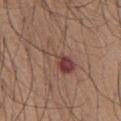This lesion was catalogued during total-body skin photography and was not selected for biopsy.
Imaged with white-light lighting.
The recorded lesion diameter is about 5 mm.
The lesion is on the chest.
The patient is a male in their mid-60s.
A 15 mm close-up tile from a total-body photography series done for melanoma screening.